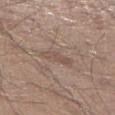subject = male, in their 30s
image-analysis metrics = an average lesion color of about L≈51 a*≈16 b*≈24 (CIELAB), a lesion–skin lightness drop of about 7, and a normalized lesion–skin contrast near 5; an automated nevus-likeness rating near 0 out of 100 and a detector confidence of about 85 out of 100 that the crop contains a lesion
tile lighting = white-light illumination
size = ≈4 mm
imaging modality = ~15 mm tile from a whole-body skin photo
location = the right lower leg